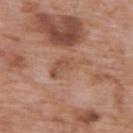biopsy status: imaged on a skin check; not biopsied
size: about 5.5 mm
illumination: white-light
automated metrics: a lesion area of about 8.5 mm² and an outline eccentricity of about 0.9 (0 = round, 1 = elongated)
patient: male, roughly 60 years of age
imaging modality: ~15 mm crop, total-body skin-cancer survey
site: the upper back